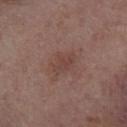notes: catalogued during a skin exam; not biopsied | lighting: white-light illumination | imaging modality: 15 mm crop, total-body photography | location: the head or neck | automated lesion analysis: an area of roughly 6.5 mm², a shape eccentricity near 0.75, and a shape-asymmetry score of about 0.25 (0 = symmetric); a within-lesion color-variation index near 2/10 and radial color variation of about 0.5 | subject: female, about 85 years old or older | lesion diameter: ≈3.5 mm.